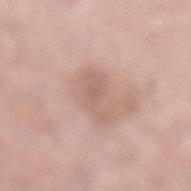biopsy status: no biopsy performed (imaged during a skin exam)
image: total-body-photography crop, ~15 mm field of view
diameter: about 5 mm
location: the left lower leg
lighting: white-light illumination
image-analysis metrics: an average lesion color of about L≈61 a*≈19 b*≈25 (CIELAB), roughly 8 lightness units darker than nearby skin, and a lesion-to-skin contrast of about 5 (normalized; higher = more distinct)
patient: female, in their mid-60s